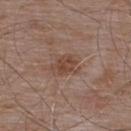Q: Is there a histopathology result?
A: total-body-photography surveillance lesion; no biopsy
Q: Lesion size?
A: about 3 mm
Q: What is the imaging modality?
A: ~15 mm crop, total-body skin-cancer survey
Q: Who is the patient?
A: male, aged around 55
Q: What is the anatomic site?
A: the upper back